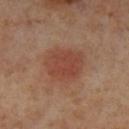<case>
  <biopsy_status>not biopsied; imaged during a skin examination</biopsy_status>
  <patient>
    <sex>female</sex>
    <age_approx>55</age_approx>
  </patient>
  <lighting>cross-polarized</lighting>
  <image>
    <source>total-body photography crop</source>
    <field_of_view_mm>15</field_of_view_mm>
  </image>
  <lesion_size>
    <long_diameter_mm_approx>4.5</long_diameter_mm_approx>
  </lesion_size>
  <site>left lower leg</site>
</case>